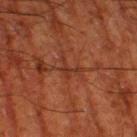No biopsy was performed on this lesion — it was imaged during a full skin examination and was not determined to be concerning. This image is a 15 mm lesion crop taken from a total-body photograph. The subject is a male aged around 80. The lesion is on the leg.